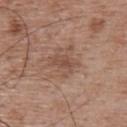  biopsy_status: not biopsied; imaged during a skin examination
  lighting: white-light
  lesion_size:
    long_diameter_mm_approx: 3.5
  automated_metrics:
    cielab_L: 49
    cielab_a: 19
    cielab_b: 27
    vs_skin_darker_L: 8.0
    border_irregularity_0_10: 5.0
    color_variation_0_10: 2.0
    peripheral_color_asymmetry: 0.5
  image:
    source: total-body photography crop
    field_of_view_mm: 15
  patient:
    sex: male
    age_approx: 50
  site: upper back